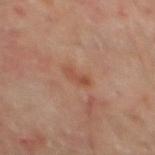| feature | finding |
|---|---|
| notes | imaged on a skin check; not biopsied |